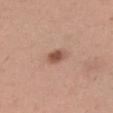workup — catalogued during a skin exam; not biopsied | patient — female, roughly 30 years of age | site — the right thigh | image source — 15 mm crop, total-body photography.